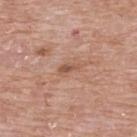Recorded during total-body skin imaging; not selected for excision or biopsy. Longest diameter approximately 2.5 mm. Automated tile analysis of the lesion measured an outline eccentricity of about 0.9 (0 = round, 1 = elongated) and two-axis asymmetry of about 0.3. And it measured a lesion-detection confidence of about 100/100. Cropped from a whole-body photographic skin survey; the tile spans about 15 mm. A female patient aged around 65. The lesion is on the chest.